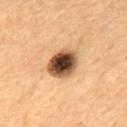<record>
<biopsy_status>not biopsied; imaged during a skin examination</biopsy_status>
<site>mid back</site>
<patient>
  <sex>male</sex>
  <age_approx>55</age_approx>
</patient>
<image>
  <source>total-body photography crop</source>
  <field_of_view_mm>15</field_of_view_mm>
</image>
</record>